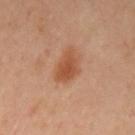Cropped from a whole-body photographic skin survey; the tile spans about 15 mm.
The lesion is on the left upper arm.
The tile uses cross-polarized illumination.
The recorded lesion diameter is about 4 mm.
The patient is a female approximately 40 years of age.
Automated tile analysis of the lesion measured a lesion area of about 7.5 mm², a shape eccentricity near 0.75, and a shape-asymmetry score of about 0.35 (0 = symmetric). The software also gave a border-irregularity index near 3.5/10, a within-lesion color-variation index near 2.5/10, and a peripheral color-asymmetry measure near 0.5. And it measured an automated nevus-likeness rating near 90 out of 100 and a detector confidence of about 100 out of 100 that the crop contains a lesion.